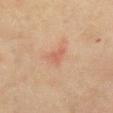Clinical impression: No biopsy was performed on this lesion — it was imaged during a full skin examination and was not determined to be concerning. Context: This is a cross-polarized tile. A roughly 15 mm field-of-view crop from a total-body skin photograph. From the chest. An algorithmic analysis of the crop reported an eccentricity of roughly 0.7 and a shape-asymmetry score of about 0.5 (0 = symmetric). And it measured an average lesion color of about L≈52 a*≈21 b*≈29 (CIELAB) and roughly 6 lightness units darker than nearby skin. It also reported a detector confidence of about 100 out of 100 that the crop contains a lesion. The lesion's longest dimension is about 3 mm. A female subject, aged approximately 70.